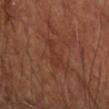biopsy status = total-body-photography surveillance lesion; no biopsy.